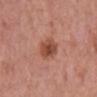Notes:
– follow-up — catalogued during a skin exam; not biopsied
– image — 15 mm crop, total-body photography
– subject — female, in their 50s
– location — the left lower leg